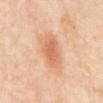  biopsy_status: not biopsied; imaged during a skin examination
  lighting: cross-polarized
  lesion_size:
    long_diameter_mm_approx: 4.0
  site: abdomen
  patient:
    sex: female
    age_approx: 50
  image:
    source: total-body photography crop
    field_of_view_mm: 15